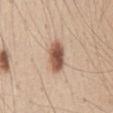Cropped from a total-body skin-imaging series; the visible field is about 15 mm.
Imaged with white-light lighting.
The lesion is located on the front of the torso.
The lesion-visualizer software estimated an area of roughly 7.5 mm², an eccentricity of roughly 0.8, and a symmetry-axis asymmetry near 0.15. The software also gave an average lesion color of about L≈54 a*≈21 b*≈29 (CIELAB), about 17 CIELAB-L* units darker than the surrounding skin, and a normalized lesion–skin contrast near 11. The software also gave a border-irregularity index near 1.5/10, internal color variation of about 5 on a 0–10 scale, and a peripheral color-asymmetry measure near 1.5. And it measured an automated nevus-likeness rating near 100 out of 100.
The recorded lesion diameter is about 4 mm.
A male subject, about 45 years old.